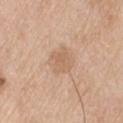notes = total-body-photography surveillance lesion; no biopsy
patient = male, approximately 75 years of age
acquisition = ~15 mm crop, total-body skin-cancer survey
lighting = white-light illumination
site = the arm